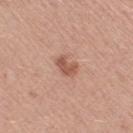Clinical impression: Imaged during a routine full-body skin examination; the lesion was not biopsied and no histopathology is available. Acquisition and patient details: Captured under white-light illumination. The total-body-photography lesion software estimated a footprint of about 4 mm² and an eccentricity of roughly 0.75. And it measured a mean CIELAB color near L≈54 a*≈23 b*≈29, roughly 11 lightness units darker than nearby skin, and a lesion-to-skin contrast of about 7.5 (normalized; higher = more distinct). The software also gave a border-irregularity index near 3.5/10 and internal color variation of about 1 on a 0–10 scale. And it measured a classifier nevus-likeness of about 60/100. On the leg. The subject is a female in their mid-50s. A close-up tile cropped from a whole-body skin photograph, about 15 mm across.